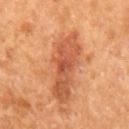Captured during whole-body skin photography for melanoma surveillance; the lesion was not biopsied. Located on the mid back. The lesion's longest dimension is about 2.5 mm. Cropped from a total-body skin-imaging series; the visible field is about 15 mm. The patient is a male in their mid-60s. Imaged with cross-polarized lighting.